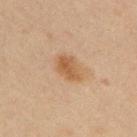• biopsy status — catalogued during a skin exam; not biopsied
• lesion diameter — ~3.5 mm (longest diameter)
• acquisition — ~15 mm crop, total-body skin-cancer survey
• patient — male, about 35 years old
• location — the upper back